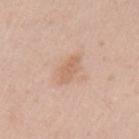Notes:
• image source — 15 mm crop, total-body photography
• location — the left upper arm
• patient — female, aged approximately 40
• size — about 4 mm
• lighting — white-light